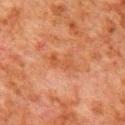biopsy status: total-body-photography surveillance lesion; no biopsy | site: the right upper arm | image source: 15 mm crop, total-body photography | patient: male, approximately 80 years of age | TBP lesion metrics: an area of roughly 5 mm², an outline eccentricity of about 0.85 (0 = round, 1 = elongated), and a shape-asymmetry score of about 0.4 (0 = symmetric); a lesion color around L≈43 a*≈23 b*≈32 in CIELAB, about 5 CIELAB-L* units darker than the surrounding skin, and a normalized lesion–skin contrast near 5; a border-irregularity index near 5/10, a within-lesion color-variation index near 2/10, and radial color variation of about 0.5; a nevus-likeness score of about 0/100 and a detector confidence of about 100 out of 100 that the crop contains a lesion | diameter: ~3.5 mm (longest diameter).